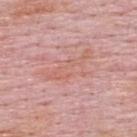{
  "biopsy_status": "not biopsied; imaged during a skin examination",
  "image": {
    "source": "total-body photography crop",
    "field_of_view_mm": 15
  },
  "lighting": "white-light",
  "patient": {
    "sex": "male",
    "age_approx": 65
  },
  "lesion_size": {
    "long_diameter_mm_approx": 6.0
  },
  "site": "upper back"
}